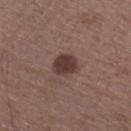* lesion size · about 3 mm
* acquisition · ~15 mm crop, total-body skin-cancer survey
* site · the right lower leg
* automated lesion analysis · a footprint of about 7 mm² and a shape-asymmetry score of about 0.15 (0 = symmetric); a mean CIELAB color near L≈37 a*≈18 b*≈19, roughly 11 lightness units darker than nearby skin, and a normalized lesion–skin contrast near 9.5; border irregularity of about 1.5 on a 0–10 scale and internal color variation of about 2 on a 0–10 scale; a classifier nevus-likeness of about 85/100 and lesion-presence confidence of about 100/100
* subject · male, about 65 years old
* illumination · white-light